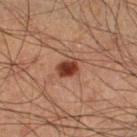Imaged during a routine full-body skin examination; the lesion was not biopsied and no histopathology is available.
Automated tile analysis of the lesion measured a border-irregularity rating of about 2/10, a within-lesion color-variation index near 4.5/10, and a peripheral color-asymmetry measure near 1.5.
This is a cross-polarized tile.
From the leg.
A male patient, approximately 60 years of age.
About 2.5 mm across.
A 15 mm crop from a total-body photograph taken for skin-cancer surveillance.